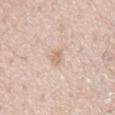| key | value |
|---|---|
| imaging modality | ~15 mm crop, total-body skin-cancer survey |
| site | the mid back |
| automated metrics | a lesion area of about 2.5 mm²; a lesion color around L≈68 a*≈17 b*≈30 in CIELAB, about 8 CIELAB-L* units darker than the surrounding skin, and a lesion-to-skin contrast of about 5.5 (normalized; higher = more distinct); a border-irregularity index near 4/10, internal color variation of about 0 on a 0–10 scale, and a peripheral color-asymmetry measure near 0; a nevus-likeness score of about 0/100 and lesion-presence confidence of about 100/100 |
| subject | male, aged 78 to 82 |